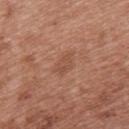| field | value |
|---|---|
| notes | imaged on a skin check; not biopsied |
| illumination | white-light illumination |
| image | ~15 mm crop, total-body skin-cancer survey |
| anatomic site | the upper back |
| patient | female, approximately 40 years of age |